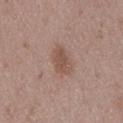Notes:
* biopsy status: no biopsy performed (imaged during a skin exam)
* illumination: white-light illumination
* location: the right thigh
* image: total-body-photography crop, ~15 mm field of view
* lesion size: ≈3.5 mm
* subject: male, about 50 years old